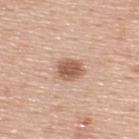- biopsy status · imaged on a skin check; not biopsied
- patient · male, roughly 50 years of age
- image · ~15 mm crop, total-body skin-cancer survey
- site · the upper back
- TBP lesion metrics · a mean CIELAB color near L≈58 a*≈21 b*≈30, roughly 14 lightness units darker than nearby skin, and a lesion-to-skin contrast of about 8.5 (normalized; higher = more distinct); a nevus-likeness score of about 95/100 and a detector confidence of about 100 out of 100 that the crop contains a lesion